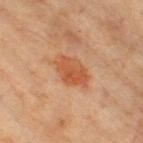{"patient": {"sex": "male", "age_approx": 55}, "automated_metrics": {"area_mm2_approx": 9.0, "eccentricity": 0.8, "shape_asymmetry": 0.2, "cielab_L": 44, "cielab_a": 22, "cielab_b": 32, "vs_skin_darker_L": 8.0, "vs_skin_contrast_norm": 7.5, "border_irregularity_0_10": 2.0, "color_variation_0_10": 3.0, "peripheral_color_asymmetry": 1.0, "nevus_likeness_0_100": 85}, "lighting": "cross-polarized", "image": {"source": "total-body photography crop", "field_of_view_mm": 15}, "lesion_size": {"long_diameter_mm_approx": 4.5}, "site": "front of the torso"}